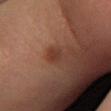Acquisition and patient details: A female subject, in their mid-20s. Imaged with cross-polarized lighting. A 15 mm close-up extracted from a 3D total-body photography capture. Automated tile analysis of the lesion measured an area of roughly 3 mm², an outline eccentricity of about 0.3 (0 = round, 1 = elongated), and a shape-asymmetry score of about 0.25 (0 = symmetric). The analysis additionally found a mean CIELAB color near L≈34 a*≈22 b*≈27 and a normalized border contrast of about 6.5. The software also gave peripheral color asymmetry of about 0.5. The software also gave a classifier nevus-likeness of about 40/100 and lesion-presence confidence of about 100/100. The lesion's longest dimension is about 2 mm. The lesion is located on the left lower leg.